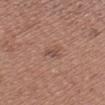Clinical impression: The lesion was photographed on a routine skin check and not biopsied; there is no pathology result. Context: The patient is a male approximately 35 years of age. About 2.5 mm across. The total-body-photography lesion software estimated an average lesion color of about L≈49 a*≈20 b*≈25 (CIELAB), roughly 8 lightness units darker than nearby skin, and a normalized border contrast of about 6. The software also gave border irregularity of about 5 on a 0–10 scale, a within-lesion color-variation index near 0/10, and radial color variation of about 0. From the head or neck. A 15 mm crop from a total-body photograph taken for skin-cancer surveillance. Imaged with white-light lighting.